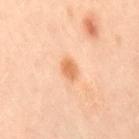Assessment: This lesion was catalogued during total-body skin photography and was not selected for biopsy. Background: A female patient roughly 40 years of age. Located on the leg. This is a cross-polarized tile. Cropped from a total-body skin-imaging series; the visible field is about 15 mm.